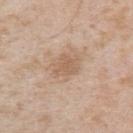workup=total-body-photography surveillance lesion; no biopsy | anatomic site=the chest | tile lighting=white-light illumination | patient=male, approximately 55 years of age | imaging modality=~15 mm tile from a whole-body skin photo.